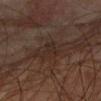Part of a total-body skin-imaging series; this lesion was reviewed on a skin check and was not flagged for biopsy. The lesion is located on the right upper arm. The recorded lesion diameter is about 3 mm. A roughly 15 mm field-of-view crop from a total-body skin photograph. Imaged with cross-polarized lighting. The lesion-visualizer software estimated a footprint of about 4.5 mm², an eccentricity of roughly 0.5, and a shape-asymmetry score of about 0.65 (0 = symmetric). The software also gave an automated nevus-likeness rating near 0 out of 100 and lesion-presence confidence of about 50/100. A male subject, in their mid- to late 70s.